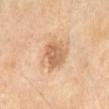<tbp_lesion>
  <lesion_size>
    <long_diameter_mm_approx>4.5</long_diameter_mm_approx>
  </lesion_size>
  <patient>
    <sex>male</sex>
    <age_approx>70</age_approx>
  </patient>
  <site>abdomen</site>
  <automated_metrics>
    <area_mm2_approx>14.0</area_mm2_approx>
    <eccentricity>0.45</eccentricity>
  </automated_metrics>
  <image>
    <source>total-body photography crop</source>
    <field_of_view_mm>15</field_of_view_mm>
  </image>
</tbp_lesion>